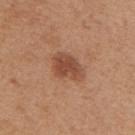The lesion was photographed on a routine skin check and not biopsied; there is no pathology result. On the back. This image is a 15 mm lesion crop taken from a total-body photograph. The subject is a female aged around 40. About 4.5 mm across. The lesion-visualizer software estimated a shape eccentricity near 0.8. The software also gave roughly 11 lightness units darker than nearby skin and a normalized border contrast of about 8. It also reported a within-lesion color-variation index near 2.5/10 and peripheral color asymmetry of about 1.